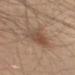No biopsy was performed on this lesion — it was imaged during a full skin examination and was not determined to be concerning. The tile uses white-light illumination. A 15 mm crop from a total-body photograph taken for skin-cancer surveillance. The subject is a male aged 58–62. On the right upper arm.